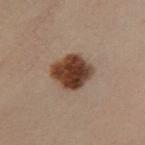Assessment: Part of a total-body skin-imaging series; this lesion was reviewed on a skin check and was not flagged for biopsy. Clinical summary: Longest diameter approximately 4.5 mm. This is a cross-polarized tile. The subject is a female in their 50s. A lesion tile, about 15 mm wide, cut from a 3D total-body photograph. The lesion is on the left lower leg.